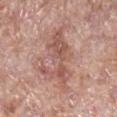Q: What is the imaging modality?
A: total-body-photography crop, ~15 mm field of view
Q: How was the tile lit?
A: white-light
Q: What are the patient's age and sex?
A: female, aged 68–72
Q: What is the anatomic site?
A: the leg